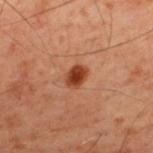<case>
  <patient>
    <sex>male</sex>
    <age_approx>60</age_approx>
  </patient>
  <site>upper back</site>
  <lesion_size>
    <long_diameter_mm_approx>2.5</long_diameter_mm_approx>
  </lesion_size>
  <lighting>cross-polarized</lighting>
  <image>
    <source>total-body photography crop</source>
    <field_of_view_mm>15</field_of_view_mm>
  </image>
</case>